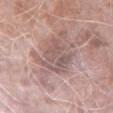biopsy status: catalogued during a skin exam; not biopsied
illumination: white-light
patient: male, aged 73–77
acquisition: total-body-photography crop, ~15 mm field of view
lesion size: ~4.5 mm (longest diameter)
body site: the arm